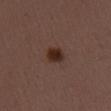Clinical impression:
No biopsy was performed on this lesion — it was imaged during a full skin examination and was not determined to be concerning.
Background:
The lesion is located on the arm. Imaged with white-light lighting. A female patient aged 48 to 52. A lesion tile, about 15 mm wide, cut from a 3D total-body photograph.